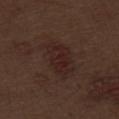• workup · no biopsy performed (imaged during a skin exam)
• acquisition · 15 mm crop, total-body photography
• body site · the abdomen
• tile lighting · white-light
• patient · male, in their 70s
• diameter · ≈5.5 mm